Cropped from a whole-body photographic skin survey; the tile spans about 15 mm, on the head or neck, the subject is a male in their mid- to late 60s — 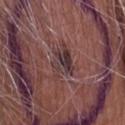Background: Longest diameter approximately 4 mm. Captured under white-light illumination. Conclusion: On biopsy, histopathology showed a dysplastic (Clark) nevus.